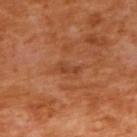The lesion-visualizer software estimated a border-irregularity rating of about 3.5/10, a within-lesion color-variation index near 0/10, and a peripheral color-asymmetry measure near 0. A 15 mm close-up extracted from a 3D total-body photography capture. Imaged with cross-polarized lighting. The lesion is located on the back. Measured at roughly 3 mm in maximum diameter. A female patient, in their mid- to late 50s.